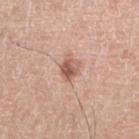The lesion was photographed on a routine skin check and not biopsied; there is no pathology result. Imaged with white-light lighting. The lesion's longest dimension is about 2.5 mm. A region of skin cropped from a whole-body photographic capture, roughly 15 mm wide. Located on the right lower leg. A male subject in their mid-60s. The lesion-visualizer software estimated a lesion area of about 4.5 mm² and a symmetry-axis asymmetry near 0.25.